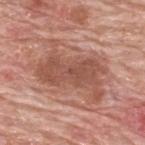follow-up: imaged on a skin check; not biopsied | lesion size: ≈7.5 mm | illumination: white-light illumination | image source: 15 mm crop, total-body photography | location: the back | patient: male, approximately 80 years of age.